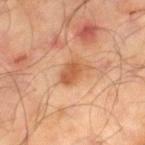Q: Was a biopsy performed?
A: total-body-photography surveillance lesion; no biopsy
Q: What are the patient's age and sex?
A: male, aged 63–67
Q: How was the tile lit?
A: cross-polarized
Q: What did automated image analysis measure?
A: a border-irregularity rating of about 3/10, a within-lesion color-variation index near 2.5/10, and radial color variation of about 1
Q: How was this image acquired?
A: ~15 mm crop, total-body skin-cancer survey
Q: What is the anatomic site?
A: the right lower leg